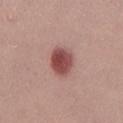Findings:
- workup — total-body-photography surveillance lesion; no biopsy
- automated metrics — an eccentricity of roughly 0.5; a border-irregularity rating of about 1.5/10, internal color variation of about 4.5 on a 0–10 scale, and peripheral color asymmetry of about 1.5; a classifier nevus-likeness of about 100/100 and lesion-presence confidence of about 100/100
- subject — female, aged 23 to 27
- anatomic site — the left lower leg
- image source — ~15 mm crop, total-body skin-cancer survey